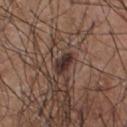| key | value |
|---|---|
| location | the chest |
| tile lighting | white-light |
| acquisition | total-body-photography crop, ~15 mm field of view |
| TBP lesion metrics | an eccentricity of roughly 0.8 |
| subject | male, approximately 55 years of age |
| diameter | ≈2.5 mm |